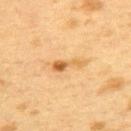{
  "biopsy_status": "not biopsied; imaged during a skin examination",
  "image": {
    "source": "total-body photography crop",
    "field_of_view_mm": 15
  },
  "lesion_size": {
    "long_diameter_mm_approx": 3.5
  },
  "site": "upper back",
  "lighting": "cross-polarized",
  "patient": {
    "sex": "female",
    "age_approx": 40
  }
}